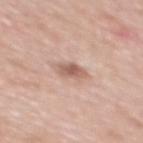Clinical impression: No biopsy was performed on this lesion — it was imaged during a full skin examination and was not determined to be concerning. Context: Automated image analysis of the tile measured a lesion area of about 4.5 mm², a shape eccentricity near 0.75, and a shape-asymmetry score of about 0.2 (0 = symmetric). And it measured a border-irregularity index near 2/10, a within-lesion color-variation index near 3.5/10, and a peripheral color-asymmetry measure near 1. A 15 mm close-up tile from a total-body photography series done for melanoma screening. The recorded lesion diameter is about 3 mm. The patient is a female aged approximately 40. The lesion is located on the back. This is a white-light tile.